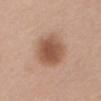biopsy_status: not biopsied; imaged during a skin examination
lesion_size:
  long_diameter_mm_approx: 5.0
site: front of the torso
patient:
  sex: female
  age_approx: 70
lighting: white-light
image:
  source: total-body photography crop
  field_of_view_mm: 15